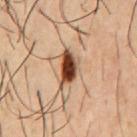The lesion was photographed on a routine skin check and not biopsied; there is no pathology result. Imaged with cross-polarized lighting. A male subject in their mid-50s. Approximately 4.5 mm at its widest. A roughly 15 mm field-of-view crop from a total-body skin photograph. The lesion is on the front of the torso.